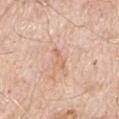Assessment:
Imaged during a routine full-body skin examination; the lesion was not biopsied and no histopathology is available.
Context:
A 15 mm close-up tile from a total-body photography series done for melanoma screening. The tile uses white-light illumination. A male patient aged 68–72. Longest diameter approximately 3 mm. The lesion is located on the right upper arm.